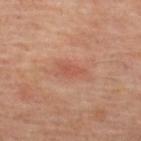Impression:
No biopsy was performed on this lesion — it was imaged during a full skin examination and was not determined to be concerning.
Background:
On the upper back. A roughly 15 mm field-of-view crop from a total-body skin photograph. A male subject, aged approximately 65.